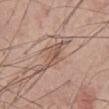Impression: The lesion was photographed on a routine skin check and not biopsied; there is no pathology result. Image and clinical context: The lesion is located on the abdomen. A lesion tile, about 15 mm wide, cut from a 3D total-body photograph. A male subject roughly 60 years of age. The total-body-photography lesion software estimated a mean CIELAB color near L≈54 a*≈18 b*≈26, roughly 8 lightness units darker than nearby skin, and a normalized border contrast of about 6. The software also gave border irregularity of about 3.5 on a 0–10 scale, a color-variation rating of about 3/10, and radial color variation of about 1. It also reported a classifier nevus-likeness of about 30/100 and lesion-presence confidence of about 60/100. The lesion's longest dimension is about 3 mm.